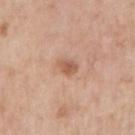Part of a total-body skin-imaging series; this lesion was reviewed on a skin check and was not flagged for biopsy.
On the right upper arm.
Automated tile analysis of the lesion measured a within-lesion color-variation index near 2/10 and radial color variation of about 0.5. The analysis additionally found lesion-presence confidence of about 100/100.
A roughly 15 mm field-of-view crop from a total-body skin photograph.
A male patient about 75 years old.
Captured under white-light illumination.
The recorded lesion diameter is about 3 mm.